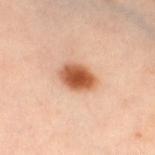The lesion is located on the left thigh.
The tile uses cross-polarized illumination.
Longest diameter approximately 4 mm.
The subject is a female aged approximately 40.
A 15 mm close-up extracted from a 3D total-body photography capture.